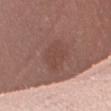The lesion was photographed on a routine skin check and not biopsied; there is no pathology result.
About 4 mm across.
The patient is a female aged 53 to 57.
The tile uses white-light illumination.
This image is a 15 mm lesion crop taken from a total-body photograph.
From the left thigh.